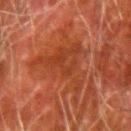| feature | finding |
|---|---|
| biopsy status | imaged on a skin check; not biopsied |
| image source | ~15 mm tile from a whole-body skin photo |
| illumination | cross-polarized |
| site | the right upper arm |
| subject | male, aged around 80 |
| lesion diameter | ~8.5 mm (longest diameter) |